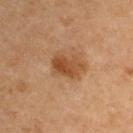No biopsy was performed on this lesion — it was imaged during a full skin examination and was not determined to be concerning.
Captured under cross-polarized illumination.
A lesion tile, about 15 mm wide, cut from a 3D total-body photograph.
The recorded lesion diameter is about 4 mm.
A male subject, aged 68 to 72.
The lesion is on the left upper arm.
An algorithmic analysis of the crop reported a border-irregularity index near 3/10, a within-lesion color-variation index near 4/10, and peripheral color asymmetry of about 1.5.